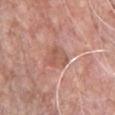Q: Is there a histopathology result?
A: imaged on a skin check; not biopsied
Q: Patient demographics?
A: male, aged approximately 50
Q: What is the imaging modality?
A: total-body-photography crop, ~15 mm field of view
Q: What is the anatomic site?
A: the chest